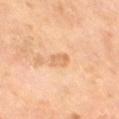Acquisition and patient details: Approximately 2.5 mm at its widest. Captured under cross-polarized illumination. The subject is a male aged around 65. Located on the leg. A 15 mm close-up tile from a total-body photography series done for melanoma screening.